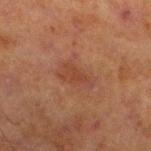Impression: Imaged during a routine full-body skin examination; the lesion was not biopsied and no histopathology is available. Clinical summary: A male patient, roughly 70 years of age. A 15 mm close-up tile from a total-body photography series done for melanoma screening. The lesion is located on the left lower leg. This is a cross-polarized tile. Automated image analysis of the tile measured a footprint of about 6.5 mm², an eccentricity of roughly 0.85, and two-axis asymmetry of about 0.3. The analysis additionally found an average lesion color of about L≈36 a*≈21 b*≈27 (CIELAB), about 6 CIELAB-L* units darker than the surrounding skin, and a normalized border contrast of about 5.5. And it measured a classifier nevus-likeness of about 5/100 and lesion-presence confidence of about 100/100. The recorded lesion diameter is about 4 mm.